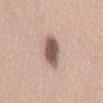{"lesion_size": {"long_diameter_mm_approx": 4.0}, "lighting": "white-light", "site": "lower back", "image": {"source": "total-body photography crop", "field_of_view_mm": 15}, "automated_metrics": {"area_mm2_approx": 8.5, "eccentricity": 0.85, "shape_asymmetry": 0.2, "border_irregularity_0_10": 2.0, "nevus_likeness_0_100": 75, "lesion_detection_confidence_0_100": 100}, "patient": {"sex": "female", "age_approx": 40}}